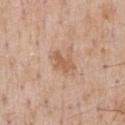The lesion was photographed on a routine skin check and not biopsied; there is no pathology result. A male subject, aged 53 to 57. Located on the chest. Cropped from a whole-body photographic skin survey; the tile spans about 15 mm.